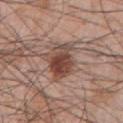{"biopsy_status": "not biopsied; imaged during a skin examination", "lighting": "white-light", "image": {"source": "total-body photography crop", "field_of_view_mm": 15}, "patient": {"sex": "male", "age_approx": 70}, "automated_metrics": {"area_mm2_approx": 12.0, "eccentricity": 0.7, "shape_asymmetry": 0.2, "border_irregularity_0_10": 3.0, "color_variation_0_10": 7.0, "nevus_likeness_0_100": 95, "lesion_detection_confidence_0_100": 100}, "lesion_size": {"long_diameter_mm_approx": 4.5}, "site": "front of the torso"}